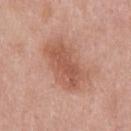No biopsy was performed on this lesion — it was imaged during a full skin examination and was not determined to be concerning.
A male subject, approximately 55 years of age.
From the front of the torso.
A lesion tile, about 15 mm wide, cut from a 3D total-body photograph.